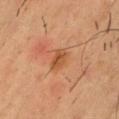Imaged during a routine full-body skin examination; the lesion was not biopsied and no histopathology is available. From the front of the torso. A 15 mm close-up extracted from a 3D total-body photography capture. About 3 mm across. A male subject aged approximately 35.